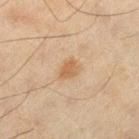{"biopsy_status": "not biopsied; imaged during a skin examination", "site": "left thigh", "image": {"source": "total-body photography crop", "field_of_view_mm": 15}, "patient": {"sex": "male", "age_approx": 45}}